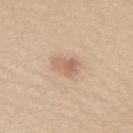Q: Was a biopsy performed?
A: imaged on a skin check; not biopsied
Q: Where on the body is the lesion?
A: the upper back
Q: Who is the patient?
A: female, in their mid-40s
Q: How was this image acquired?
A: 15 mm crop, total-body photography